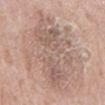Context: The subject is a male in their 60s. On the back. A close-up tile cropped from a whole-body skin photograph, about 15 mm across. The lesion-visualizer software estimated an area of roughly 44 mm² and a symmetry-axis asymmetry near 0.3. The software also gave a nevus-likeness score of about 0/100. About 13 mm across.